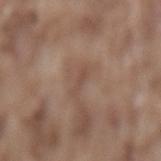Impression:
The lesion was photographed on a routine skin check and not biopsied; there is no pathology result.
Clinical summary:
From the lower back. A male patient about 75 years old. Cropped from a total-body skin-imaging series; the visible field is about 15 mm. Automated tile analysis of the lesion measured an outline eccentricity of about 0.95 (0 = round, 1 = elongated). It also reported roughly 6 lightness units darker than nearby skin.